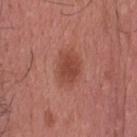The recorded lesion diameter is about 4 mm. From the head or neck. The patient is a male roughly 35 years of age. A 15 mm close-up extracted from a 3D total-body photography capture.